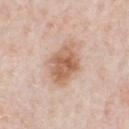Part of a total-body skin-imaging series; this lesion was reviewed on a skin check and was not flagged for biopsy.
An algorithmic analysis of the crop reported a border-irregularity rating of about 2/10, a color-variation rating of about 5/10, and a peripheral color-asymmetry measure near 1.5.
A roughly 15 mm field-of-view crop from a total-body skin photograph.
The lesion's longest dimension is about 5 mm.
Located on the chest.
A male patient, aged 58–62.